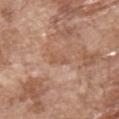| field | value |
|---|---|
| workup | catalogued during a skin exam; not biopsied |
| imaging modality | ~15 mm crop, total-body skin-cancer survey |
| TBP lesion metrics | a mean CIELAB color near L≈55 a*≈22 b*≈31, a lesion–skin lightness drop of about 6, and a normalized border contrast of about 5; a classifier nevus-likeness of about 0/100 and a detector confidence of about 75 out of 100 that the crop contains a lesion |
| body site | the chest |
| patient | male, aged 68–72 |
| illumination | white-light |